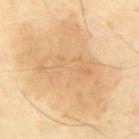The lesion was photographed on a routine skin check and not biopsied; there is no pathology result.
The lesion is located on the mid back.
A close-up tile cropped from a whole-body skin photograph, about 15 mm across.
Captured under cross-polarized illumination.
A male subject, aged approximately 65.
Longest diameter approximately 12.5 mm.